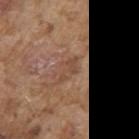Assessment:
Imaged during a routine full-body skin examination; the lesion was not biopsied and no histopathology is available.
Context:
A 15 mm crop from a total-body photograph taken for skin-cancer surveillance. The lesion is on the right upper arm. A male patient, aged 73 to 77. Captured under white-light illumination. Measured at roughly 3.5 mm in maximum diameter.